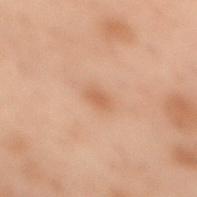– subject: female, in their mid- to late 50s
– lighting: cross-polarized illumination
– image source: ~15 mm crop, total-body skin-cancer survey
– diameter: about 2.5 mm
– location: the upper back
– TBP lesion metrics: a shape eccentricity near 0.7 and a shape-asymmetry score of about 0.15 (0 = symmetric); roughly 6 lightness units darker than nearby skin and a lesion-to-skin contrast of about 5 (normalized; higher = more distinct); internal color variation of about 1.5 on a 0–10 scale and peripheral color asymmetry of about 0.5; an automated nevus-likeness rating near 50 out of 100 and a detector confidence of about 100 out of 100 that the crop contains a lesion